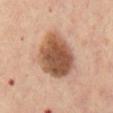Findings:
- follow-up — catalogued during a skin exam; not biopsied